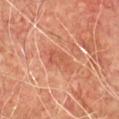This lesion was catalogued during total-body skin photography and was not selected for biopsy. Measured at roughly 3.5 mm in maximum diameter. An algorithmic analysis of the crop reported an average lesion color of about L≈46 a*≈24 b*≈30 (CIELAB), a lesion–skin lightness drop of about 6, and a lesion-to-skin contrast of about 5 (normalized; higher = more distinct). And it measured lesion-presence confidence of about 100/100. A 15 mm crop from a total-body photograph taken for skin-cancer surveillance. On the chest. This is a cross-polarized tile. A male patient in their mid- to late 70s.